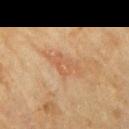Clinical impression: The lesion was tiled from a total-body skin photograph and was not biopsied.